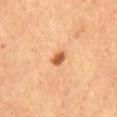{
  "biopsy_status": "not biopsied; imaged during a skin examination",
  "site": "abdomen",
  "patient": {
    "sex": "female"
  },
  "image": {
    "source": "total-body photography crop",
    "field_of_view_mm": 15
  },
  "lesion_size": {
    "long_diameter_mm_approx": 2.0
  }
}